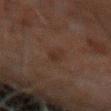follow-up = no biopsy performed (imaged during a skin exam)
imaging modality = ~15 mm crop, total-body skin-cancer survey
patient = male, aged 58–62
location = the arm
tile lighting = cross-polarized illumination